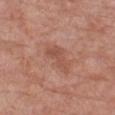This lesion was catalogued during total-body skin photography and was not selected for biopsy.
A 15 mm crop from a total-body photograph taken for skin-cancer surveillance.
A male subject, aged approximately 80.
The lesion is on the right lower leg.